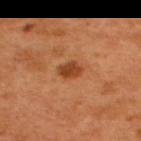Q: Is there a histopathology result?
A: catalogued during a skin exam; not biopsied
Q: Patient demographics?
A: male, aged 48 to 52
Q: What is the imaging modality?
A: ~15 mm crop, total-body skin-cancer survey
Q: Lesion location?
A: the upper back
Q: What is the lesion's diameter?
A: ~3 mm (longest diameter)
Q: Illumination type?
A: cross-polarized illumination